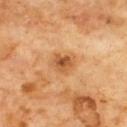Notes:
* TBP lesion metrics — a nevus-likeness score of about 10/100
* image source — ~15 mm crop, total-body skin-cancer survey
* location — the chest
* illumination — cross-polarized illumination
* diameter — ≈3 mm
* patient — male, approximately 60 years of age